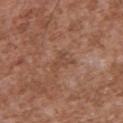Captured during whole-body skin photography for melanoma surveillance; the lesion was not biopsied.
A 15 mm crop from a total-body photograph taken for skin-cancer surveillance.
On the front of the torso.
The subject is a male approximately 45 years of age.